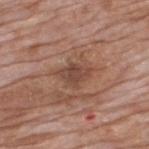The lesion was photographed on a routine skin check and not biopsied; there is no pathology result. A male subject aged around 60. A 15 mm crop from a total-body photograph taken for skin-cancer surveillance. Measured at roughly 3.5 mm in maximum diameter. Automated image analysis of the tile measured an average lesion color of about L≈45 a*≈21 b*≈27 (CIELAB), about 9 CIELAB-L* units darker than the surrounding skin, and a normalized lesion–skin contrast near 7. Located on the upper back.